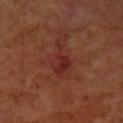The lesion was photographed on a routine skin check and not biopsied; there is no pathology result.
Automated image analysis of the tile measured an area of roughly 8 mm², an eccentricity of roughly 0.85, and a symmetry-axis asymmetry near 0.5. And it measured a lesion-to-skin contrast of about 7 (normalized; higher = more distinct).
The lesion is on the right forearm.
The subject is a female approximately 50 years of age.
A 15 mm close-up tile from a total-body photography series done for melanoma screening.
Imaged with cross-polarized lighting.
Longest diameter approximately 5 mm.